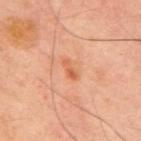Captured during whole-body skin photography for melanoma surveillance; the lesion was not biopsied. Located on the upper back. The subject is a male approximately 50 years of age. The recorded lesion diameter is about 2.5 mm. The tile uses cross-polarized illumination. A region of skin cropped from a whole-body photographic capture, roughly 15 mm wide.